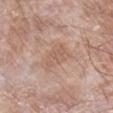Q: Was this lesion biopsied?
A: total-body-photography surveillance lesion; no biopsy
Q: Patient demographics?
A: male, about 75 years old
Q: What did automated image analysis measure?
A: a lesion area of about 8.5 mm², an outline eccentricity of about 0.75 (0 = round, 1 = elongated), and a shape-asymmetry score of about 0.4 (0 = symmetric); a lesion color around L≈59 a*≈18 b*≈28 in CIELAB and a lesion–skin lightness drop of about 7; a border-irregularity rating of about 4/10 and radial color variation of about 1
Q: What is the anatomic site?
A: the right lower leg
Q: Lesion size?
A: ~4 mm (longest diameter)
Q: How was the tile lit?
A: white-light
Q: What is the imaging modality?
A: total-body-photography crop, ~15 mm field of view